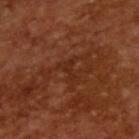{"biopsy_status": "not biopsied; imaged during a skin examination", "patient": {"sex": "male", "age_approx": 65}, "image": {"source": "total-body photography crop", "field_of_view_mm": 15}}